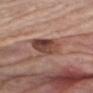Assessment: Part of a total-body skin-imaging series; this lesion was reviewed on a skin check and was not flagged for biopsy. Image and clinical context: A 15 mm close-up tile from a total-body photography series done for melanoma screening. Located on the left upper arm. This is a white-light tile. A male subject, aged 73 to 77. The total-body-photography lesion software estimated an area of roughly 12 mm², a shape eccentricity near 0.35, and a shape-asymmetry score of about 0.25 (0 = symmetric). The software also gave a lesion color around L≈45 a*≈21 b*≈25 in CIELAB, a lesion–skin lightness drop of about 13, and a normalized lesion–skin contrast near 9.5.